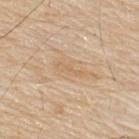Impression: This lesion was catalogued during total-body skin photography and was not selected for biopsy. Clinical summary: On the back. A male patient approximately 80 years of age. The recorded lesion diameter is about 4 mm. Imaged with white-light lighting. Cropped from a total-body skin-imaging series; the visible field is about 15 mm.